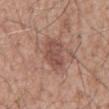| feature | finding |
|---|---|
| biopsy status | no biopsy performed (imaged during a skin exam) |
| tile lighting | white-light |
| imaging modality | ~15 mm tile from a whole-body skin photo |
| anatomic site | the mid back |
| patient | male, approximately 60 years of age |